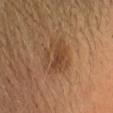Captured under cross-polarized illumination. This image is a 15 mm lesion crop taken from a total-body photograph. The lesion's longest dimension is about 4.5 mm. A female patient roughly 50 years of age.